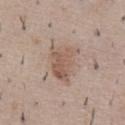Impression:
The lesion was photographed on a routine skin check and not biopsied; there is no pathology result.
Acquisition and patient details:
The total-body-photography lesion software estimated about 9 CIELAB-L* units darker than the surrounding skin. The lesion is located on the chest. The subject is a male approximately 50 years of age. Imaged with white-light lighting. A region of skin cropped from a whole-body photographic capture, roughly 15 mm wide.